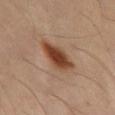A roughly 15 mm field-of-view crop from a total-body skin photograph. Located on the mid back. The subject is a male aged 53–57.